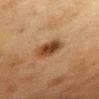follow-up: total-body-photography surveillance lesion; no biopsy
acquisition: 15 mm crop, total-body photography
image-analysis metrics: border irregularity of about 1.5 on a 0–10 scale, a within-lesion color-variation index near 5/10, and peripheral color asymmetry of about 1.5
illumination: cross-polarized illumination
location: the chest
subject: female, in their mid- to late 50s
lesion diameter: ~4 mm (longest diameter)